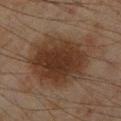Part of a total-body skin-imaging series; this lesion was reviewed on a skin check and was not flagged for biopsy. A male patient, aged around 45. The tile uses cross-polarized illumination. An algorithmic analysis of the crop reported an area of roughly 44 mm² and two-axis asymmetry of about 0.2. It also reported a lesion–skin lightness drop of about 9 and a lesion-to-skin contrast of about 9 (normalized; higher = more distinct). The analysis additionally found a classifier nevus-likeness of about 70/100 and lesion-presence confidence of about 100/100. The lesion is located on the leg. A 15 mm close-up extracted from a 3D total-body photography capture. Approximately 8.5 mm at its widest.